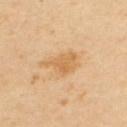Acquisition and patient details:
Located on the back. The patient is a male aged 53–57. A 15 mm crop from a total-body photograph taken for skin-cancer surveillance.